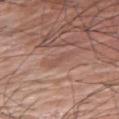Captured during whole-body skin photography for melanoma surveillance; the lesion was not biopsied.
Cropped from a total-body skin-imaging series; the visible field is about 15 mm.
A male subject aged 73 to 77.
The lesion is on the arm.